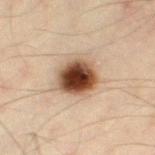<record>
<biopsy_status>not biopsied; imaged during a skin examination</biopsy_status>
<site>left thigh</site>
<automated_metrics>
  <border_irregularity_0_10>1.5</border_irregularity_0_10>
  <color_variation_0_10>8.0</color_variation_0_10>
</automated_metrics>
<image>
  <source>total-body photography crop</source>
  <field_of_view_mm>15</field_of_view_mm>
</image>
<lighting>cross-polarized</lighting>
<patient>
  <sex>male</sex>
  <age_approx>45</age_approx>
</patient>
<lesion_size>
  <long_diameter_mm_approx>4.0</long_diameter_mm_approx>
</lesion_size>
</record>